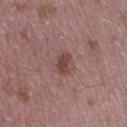follow-up: no biopsy performed (imaged during a skin exam) | lighting: white-light illumination | size: about 2.5 mm | patient: male, aged around 40 | anatomic site: the left lower leg | acquisition: 15 mm crop, total-body photography.